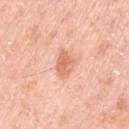The lesion was tiled from a total-body skin photograph and was not biopsied.
A male subject in their 50s.
On the left upper arm.
A lesion tile, about 15 mm wide, cut from a 3D total-body photograph.